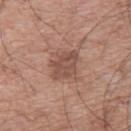A 15 mm close-up tile from a total-body photography series done for melanoma screening. An algorithmic analysis of the crop reported a footprint of about 8 mm², a shape eccentricity near 0.75, and two-axis asymmetry of about 0.2. The analysis additionally found border irregularity of about 2.5 on a 0–10 scale, internal color variation of about 3 on a 0–10 scale, and radial color variation of about 1. A male subject, aged 63 to 67. From the upper back. Measured at roughly 4 mm in maximum diameter.